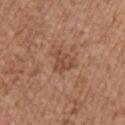Recorded during total-body skin imaging; not selected for excision or biopsy.